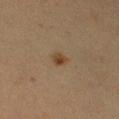Captured during whole-body skin photography for melanoma surveillance; the lesion was not biopsied. From the right upper arm. A female patient, aged around 40. Cropped from a whole-body photographic skin survey; the tile spans about 15 mm. The tile uses cross-polarized illumination. Longest diameter approximately 2 mm.